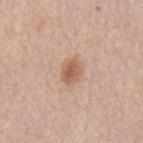• workup: total-body-photography surveillance lesion; no biopsy